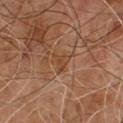Impression: Part of a total-body skin-imaging series; this lesion was reviewed on a skin check and was not flagged for biopsy. Acquisition and patient details: A male subject, aged 63–67. Cropped from a whole-body photographic skin survey; the tile spans about 15 mm.